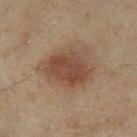Notes:
– follow-up — imaged on a skin check; not biopsied
– image source — ~15 mm crop, total-body skin-cancer survey
– tile lighting — cross-polarized illumination
– subject — female, aged 58 to 62
– automated lesion analysis — an area of roughly 21 mm², an eccentricity of roughly 0.65, and a symmetry-axis asymmetry near 0.2
– body site — the left lower leg
– lesion diameter — ≈6.5 mm